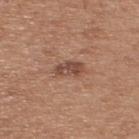Located on the upper back. The total-body-photography lesion software estimated a mean CIELAB color near L≈47 a*≈21 b*≈27 and a lesion–skin lightness drop of about 10. Imaged with white-light lighting. A male subject aged 53–57. The recorded lesion diameter is about 3 mm. Cropped from a total-body skin-imaging series; the visible field is about 15 mm.